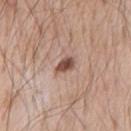Imaged during a routine full-body skin examination; the lesion was not biopsied and no histopathology is available.
Cropped from a total-body skin-imaging series; the visible field is about 15 mm.
Imaged with white-light lighting.
The lesion is on the chest.
A male subject, approximately 55 years of age.
Measured at roughly 2.5 mm in maximum diameter.